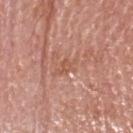biopsy status = no biopsy performed (imaged during a skin exam).